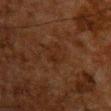| key | value |
|---|---|
| biopsy status | no biopsy performed (imaged during a skin exam) |
| acquisition | ~15 mm tile from a whole-body skin photo |
| location | the chest |
| patient | male, in their 60s |